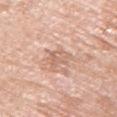A roughly 15 mm field-of-view crop from a total-body skin photograph. An algorithmic analysis of the crop reported an area of roughly 5.5 mm². The analysis additionally found a nevus-likeness score of about 0/100 and lesion-presence confidence of about 95/100. Located on the right upper arm. Approximately 3 mm at its widest. This is a white-light tile. A female subject aged 73 to 77.